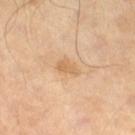Assessment: No biopsy was performed on this lesion — it was imaged during a full skin examination and was not determined to be concerning. Context: A region of skin cropped from a whole-body photographic capture, roughly 15 mm wide. A male patient, about 55 years old. Approximately 3 mm at its widest. This is a cross-polarized tile. From the right thigh.